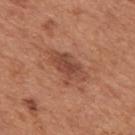Q: Was this lesion biopsied?
A: no biopsy performed (imaged during a skin exam)
Q: What kind of image is this?
A: ~15 mm crop, total-body skin-cancer survey
Q: What is the lesion's diameter?
A: ≈5 mm
Q: Where on the body is the lesion?
A: the mid back
Q: What lighting was used for the tile?
A: white-light
Q: What are the patient's age and sex?
A: male, in their mid-60s
Q: What did automated image analysis measure?
A: a lesion color around L≈47 a*≈24 b*≈30 in CIELAB, about 9 CIELAB-L* units darker than the surrounding skin, and a lesion-to-skin contrast of about 7 (normalized; higher = more distinct)